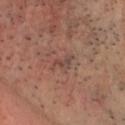The lesion was photographed on a routine skin check and not biopsied; there is no pathology result. A 15 mm close-up extracted from a 3D total-body photography capture. The lesion is located on the head or neck. The total-body-photography lesion software estimated an eccentricity of roughly 0.9 and a shape-asymmetry score of about 0.5 (0 = symmetric). Imaged with cross-polarized lighting. The patient is in their mid-50s.